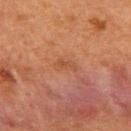Assessment: Captured during whole-body skin photography for melanoma surveillance; the lesion was not biopsied. Acquisition and patient details: A female patient, roughly 50 years of age. The lesion is on the upper back. This image is a 15 mm lesion crop taken from a total-body photograph.